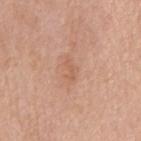{
  "biopsy_status": "not biopsied; imaged during a skin examination",
  "patient": {
    "sex": "female",
    "age_approx": 65
  },
  "image": {
    "source": "total-body photography crop",
    "field_of_view_mm": 15
  },
  "site": "mid back",
  "lesion_size": {
    "long_diameter_mm_approx": 2.5
  },
  "lighting": "white-light"
}